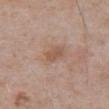Part of a total-body skin-imaging series; this lesion was reviewed on a skin check and was not flagged for biopsy.
Captured under white-light illumination.
About 2.5 mm across.
The total-body-photography lesion software estimated a lesion area of about 4 mm², a shape eccentricity near 0.7, and a shape-asymmetry score of about 0.2 (0 = symmetric). And it measured an average lesion color of about L≈54 a*≈19 b*≈29 (CIELAB), a lesion–skin lightness drop of about 8, and a normalized lesion–skin contrast near 6. And it measured a border-irregularity rating of about 2/10, a within-lesion color-variation index near 1/10, and radial color variation of about 0.5.
A male subject in their mid- to late 60s.
From the abdomen.
A region of skin cropped from a whole-body photographic capture, roughly 15 mm wide.